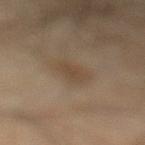- workup · imaged on a skin check; not biopsied
- automated metrics · a lesion area of about 4.5 mm², a shape eccentricity near 0.75, and a shape-asymmetry score of about 0.25 (0 = symmetric); a border-irregularity index near 2.5/10, a color-variation rating of about 1.5/10, and radial color variation of about 0.5
- lighting · cross-polarized illumination
- subject · male, in their mid-60s
- image · total-body-photography crop, ~15 mm field of view
- anatomic site · the left lower leg